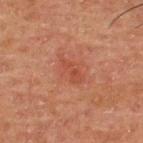This lesion was catalogued during total-body skin photography and was not selected for biopsy. The tile uses cross-polarized illumination. A 15 mm close-up tile from a total-body photography series done for melanoma screening. Measured at roughly 3.5 mm in maximum diameter. Located on the upper back. A male subject in their 50s.